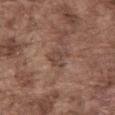No biopsy was performed on this lesion — it was imaged during a full skin examination and was not determined to be concerning.
A male patient, about 75 years old.
The lesion-visualizer software estimated a footprint of about 4 mm² and an eccentricity of roughly 0.5. The software also gave an automated nevus-likeness rating near 0 out of 100.
This image is a 15 mm lesion crop taken from a total-body photograph.
About 2.5 mm across.
The tile uses white-light illumination.
Located on the abdomen.